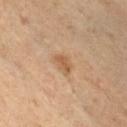Impression:
Part of a total-body skin-imaging series; this lesion was reviewed on a skin check and was not flagged for biopsy.
Image and clinical context:
The tile uses cross-polarized illumination. This image is a 15 mm lesion crop taken from a total-body photograph. The lesion-visualizer software estimated an average lesion color of about L≈56 a*≈19 b*≈36 (CIELAB), about 9 CIELAB-L* units darker than the surrounding skin, and a normalized lesion–skin contrast near 6.5. The software also gave border irregularity of about 3.5 on a 0–10 scale and peripheral color asymmetry of about 0.5. The subject is a male roughly 45 years of age. From the chest.